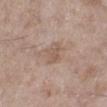Clinical impression: The lesion was photographed on a routine skin check and not biopsied; there is no pathology result. Acquisition and patient details: Approximately 2.5 mm at its widest. A 15 mm crop from a total-body photograph taken for skin-cancer surveillance. A female patient, about 60 years old. The tile uses white-light illumination. The total-body-photography lesion software estimated a lesion color around L≈56 a*≈17 b*≈26 in CIELAB and about 7 CIELAB-L* units darker than the surrounding skin. And it measured border irregularity of about 3.5 on a 0–10 scale and internal color variation of about 2 on a 0–10 scale. And it measured a classifier nevus-likeness of about 0/100 and a lesion-detection confidence of about 100/100. The lesion is located on the left lower leg.